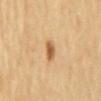Assessment: Captured during whole-body skin photography for melanoma surveillance; the lesion was not biopsied. Context: This is a cross-polarized tile. Located on the mid back. Longest diameter approximately 3 mm. The subject is a male roughly 70 years of age. A lesion tile, about 15 mm wide, cut from a 3D total-body photograph.